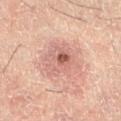follow-up: catalogued during a skin exam; not biopsied
patient: male, aged around 50
body site: the left thigh
image: total-body-photography crop, ~15 mm field of view
TBP lesion metrics: roughly 9 lightness units darker than nearby skin and a lesion-to-skin contrast of about 6 (normalized; higher = more distinct); a border-irregularity rating of about 3/10, a color-variation rating of about 7.5/10, and a peripheral color-asymmetry measure near 2.5
diameter: ~5 mm (longest diameter)